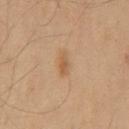• patient · male, about 60 years old
• location · the chest
• diameter · ~3 mm (longest diameter)
• illumination · cross-polarized illumination
• acquisition · ~15 mm tile from a whole-body skin photo
• automated metrics · an area of roughly 3.5 mm², a shape eccentricity near 0.9, and two-axis asymmetry of about 0.2; a lesion color around L≈52 a*≈17 b*≈33 in CIELAB, roughly 7 lightness units darker than nearby skin, and a normalized lesion–skin contrast near 6; a classifier nevus-likeness of about 55/100 and a detector confidence of about 100 out of 100 that the crop contains a lesion